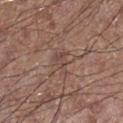This lesion was catalogued during total-body skin photography and was not selected for biopsy. A region of skin cropped from a whole-body photographic capture, roughly 15 mm wide. Approximately 3 mm at its widest. This is a white-light tile. A male patient approximately 60 years of age. The lesion is on the leg.